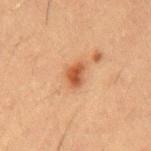• follow-up — total-body-photography surveillance lesion; no biopsy
• subject — male, approximately 55 years of age
• image — 15 mm crop, total-body photography
• TBP lesion metrics — an area of roughly 4 mm² and a shape eccentricity near 0.75; an average lesion color of about L≈47 a*≈25 b*≈35 (CIELAB), a lesion–skin lightness drop of about 12, and a normalized lesion–skin contrast near 9
• location — the chest
• lighting — cross-polarized illumination